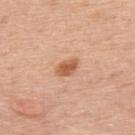{"biopsy_status": "not biopsied; imaged during a skin examination", "site": "upper back", "image": {"source": "total-body photography crop", "field_of_view_mm": 15}, "lesion_size": {"long_diameter_mm_approx": 3.0}, "lighting": "white-light", "patient": {"sex": "male", "age_approx": 75}}